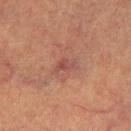biopsy_status: not biopsied; imaged during a skin examination
lighting: cross-polarized
automated_metrics:
  area_mm2_approx: 4.0
  eccentricity: 0.85
patient:
  sex: female
  age_approx: 40
lesion_size:
  long_diameter_mm_approx: 3.0
image:
  source: total-body photography crop
  field_of_view_mm: 15
site: leg